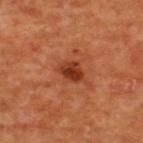notes: catalogued during a skin exam; not biopsied | imaging modality: ~15 mm crop, total-body skin-cancer survey | tile lighting: cross-polarized illumination | location: the upper back | patient: male, aged 53 to 57 | size: about 3 mm.